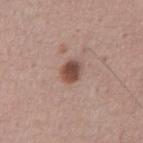The lesion was photographed on a routine skin check and not biopsied; there is no pathology result.